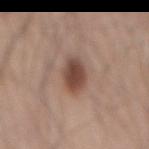Q: Is there a histopathology result?
A: imaged on a skin check; not biopsied
Q: What is the imaging modality?
A: total-body-photography crop, ~15 mm field of view
Q: Where on the body is the lesion?
A: the mid back
Q: How was the tile lit?
A: white-light illumination
Q: Patient demographics?
A: male, aged 68 to 72